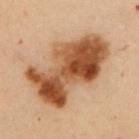Clinical impression: Part of a total-body skin-imaging series; this lesion was reviewed on a skin check and was not flagged for biopsy. Image and clinical context: The total-body-photography lesion software estimated a lesion area of about 41 mm². About 9.5 mm across. A 15 mm close-up tile from a total-body photography series done for melanoma screening. On the front of the torso. A female patient aged 48 to 52.